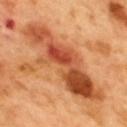- notes · no biopsy performed (imaged during a skin exam)
- tile lighting · cross-polarized illumination
- subject · male, aged 48 to 52
- lesion size · ≈12 mm
- location · the upper back
- automated metrics · an average lesion color of about L≈53 a*≈30 b*≈41 (CIELAB), roughly 14 lightness units darker than nearby skin, and a normalized lesion–skin contrast near 9.5; a classifier nevus-likeness of about 0/100
- image source · ~15 mm tile from a whole-body skin photo